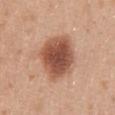No biopsy was performed on this lesion — it was imaged during a full skin examination and was not determined to be concerning.